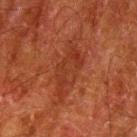Case summary:
* workup · catalogued during a skin exam; not biopsied
* body site · the left upper arm
* subject · male, aged 78 to 82
* imaging modality · 15 mm crop, total-body photography